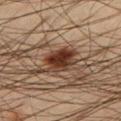Part of a total-body skin-imaging series; this lesion was reviewed on a skin check and was not flagged for biopsy. A male patient, about 45 years old. Imaged with cross-polarized lighting. Located on the left lower leg. This image is a 15 mm lesion crop taken from a total-body photograph.